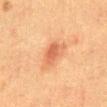This lesion was catalogued during total-body skin photography and was not selected for biopsy.
Captured under cross-polarized illumination.
The recorded lesion diameter is about 3.5 mm.
A close-up tile cropped from a whole-body skin photograph, about 15 mm across.
The subject is a male aged 48 to 52.
The total-body-photography lesion software estimated roughly 9 lightness units darker than nearby skin. The software also gave border irregularity of about 3.5 on a 0–10 scale, internal color variation of about 2.5 on a 0–10 scale, and a peripheral color-asymmetry measure near 0.5.
On the abdomen.